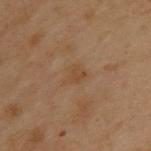No biopsy was performed on this lesion — it was imaged during a full skin examination and was not determined to be concerning.
Approximately 2.5 mm at its widest.
The total-body-photography lesion software estimated a nevus-likeness score of about 0/100 and a lesion-detection confidence of about 100/100.
A male subject aged approximately 55.
Imaged with cross-polarized lighting.
The lesion is located on the upper back.
A roughly 15 mm field-of-view crop from a total-body skin photograph.